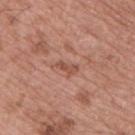<record>
<site>upper back</site>
<lighting>white-light</lighting>
<automated_metrics>
  <cielab_L>51</cielab_L>
  <cielab_a>24</cielab_a>
  <cielab_b>28</cielab_b>
  <vs_skin_darker_L>9.0</vs_skin_darker_L>
</automated_metrics>
<patient>
  <sex>male</sex>
  <age_approx>65</age_approx>
</patient>
<image>
  <source>total-body photography crop</source>
  <field_of_view_mm>15</field_of_view_mm>
</image>
</record>